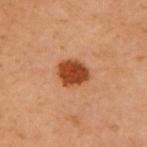workup: imaged on a skin check; not biopsied
image source: ~15 mm crop, total-body skin-cancer survey
diameter: about 3 mm
anatomic site: the chest
subject: female, aged 58 to 62
tile lighting: cross-polarized